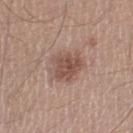workup=total-body-photography surveillance lesion; no biopsy
anatomic site=the left thigh
image=~15 mm tile from a whole-body skin photo
subject=male, approximately 45 years of age
lighting=white-light